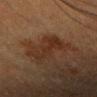The lesion was photographed on a routine skin check and not biopsied; there is no pathology result.
The lesion is on the head or neck.
The subject is a female roughly 40 years of age.
Automated image analysis of the tile measured a lesion area of about 9.5 mm², a shape eccentricity near 0.95, and a symmetry-axis asymmetry near 0.4. And it measured a lesion color around L≈25 a*≈17 b*≈25 in CIELAB and a lesion-to-skin contrast of about 7 (normalized; higher = more distinct). The software also gave a nevus-likeness score of about 0/100 and a lesion-detection confidence of about 100/100.
A close-up tile cropped from a whole-body skin photograph, about 15 mm across.
This is a cross-polarized tile.
The lesion's longest dimension is about 5.5 mm.